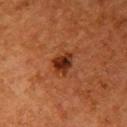notes: catalogued during a skin exam; not biopsied | illumination: cross-polarized illumination | TBP lesion metrics: a mean CIELAB color near L≈26 a*≈24 b*≈29, a lesion–skin lightness drop of about 11, and a normalized lesion–skin contrast near 10.5; a nevus-likeness score of about 90/100 and lesion-presence confidence of about 100/100 | patient: female, aged around 50 | diameter: ≈3 mm | body site: the arm | imaging modality: total-body-photography crop, ~15 mm field of view.